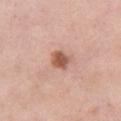Captured during whole-body skin photography for melanoma surveillance; the lesion was not biopsied. An algorithmic analysis of the crop reported a footprint of about 4.5 mm² and an outline eccentricity of about 0.65 (0 = round, 1 = elongated). And it measured an automated nevus-likeness rating near 95 out of 100 and a lesion-detection confidence of about 100/100. The tile uses white-light illumination. Located on the chest. This image is a 15 mm lesion crop taken from a total-body photograph. A female patient, approximately 65 years of age.